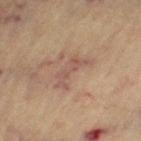The lesion was photographed on a routine skin check and not biopsied; there is no pathology result. The lesion-visualizer software estimated a footprint of about 6.5 mm², an eccentricity of roughly 0.9, and a symmetry-axis asymmetry near 0.6. The software also gave about 7 CIELAB-L* units darker than the surrounding skin. The software also gave a border-irregularity rating of about 7.5/10 and a color-variation rating of about 2/10. The analysis additionally found lesion-presence confidence of about 100/100. The subject is a female aged 63–67. Cropped from a total-body skin-imaging series; the visible field is about 15 mm. On the left thigh. This is a cross-polarized tile.